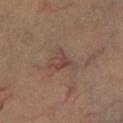The lesion was photographed on a routine skin check and not biopsied; there is no pathology result.
The recorded lesion diameter is about 3 mm.
A male patient, aged approximately 60.
The tile uses cross-polarized illumination.
A 15 mm close-up tile from a total-body photography series done for melanoma screening.
From the right lower leg.